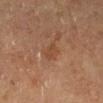Clinical impression: The lesion was tiled from a total-body skin photograph and was not biopsied. Acquisition and patient details: The subject is a male roughly 85 years of age or older. A roughly 15 mm field-of-view crop from a total-body skin photograph. The lesion is located on the right lower leg.